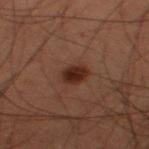<case>
<patient>
  <sex>male</sex>
  <age_approx>60</age_approx>
</patient>
<site>right thigh</site>
<image>
  <source>total-body photography crop</source>
  <field_of_view_mm>15</field_of_view_mm>
</image>
</case>